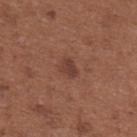workup: imaged on a skin check; not biopsied
acquisition: ~15 mm tile from a whole-body skin photo
subject: female, in their mid-60s
site: the upper back